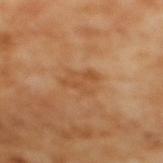Clinical impression:
Imaged during a routine full-body skin examination; the lesion was not biopsied and no histopathology is available.
Image and clinical context:
A 15 mm close-up tile from a total-body photography series done for melanoma screening. A female patient aged approximately 55. Approximately 4 mm at its widest. The tile uses cross-polarized illumination. The lesion is located on the mid back.